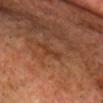biopsy status=catalogued during a skin exam; not biopsied